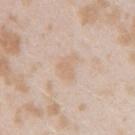Impression: The lesion was tiled from a total-body skin photograph and was not biopsied. Context: The lesion is located on the arm. Longest diameter approximately 3 mm. The total-body-photography lesion software estimated a mean CIELAB color near L≈68 a*≈15 b*≈29, a lesion–skin lightness drop of about 6, and a normalized border contrast of about 4.5. The analysis additionally found border irregularity of about 3 on a 0–10 scale, a within-lesion color-variation index near 1.5/10, and a peripheral color-asymmetry measure near 0.5. The analysis additionally found a nevus-likeness score of about 0/100. The subject is a female roughly 25 years of age. A close-up tile cropped from a whole-body skin photograph, about 15 mm across.